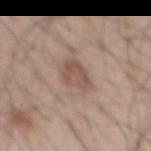This lesion was catalogued during total-body skin photography and was not selected for biopsy.
The lesion-visualizer software estimated a lesion area of about 7.5 mm² and a shape eccentricity near 0.8. The software also gave a border-irregularity index near 3/10, a within-lesion color-variation index near 3/10, and radial color variation of about 1.
A roughly 15 mm field-of-view crop from a total-body skin photograph.
Measured at roughly 4 mm in maximum diameter.
A male subject, roughly 50 years of age.
The lesion is on the mid back.